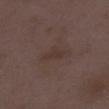<lesion>
<biopsy_status>not biopsied; imaged during a skin examination</biopsy_status>
<site>right lower leg</site>
<image>
  <source>total-body photography crop</source>
  <field_of_view_mm>15</field_of_view_mm>
</image>
<patient>
  <sex>female</sex>
  <age_approx>30</age_approx>
</patient>
</lesion>